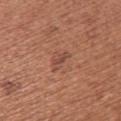patient: female, aged 48 to 52 | image-analysis metrics: a footprint of about 3.5 mm², a shape eccentricity near 0.9, and a shape-asymmetry score of about 0.4 (0 = symmetric); a lesion color around L≈48 a*≈23 b*≈28 in CIELAB, roughly 7 lightness units darker than nearby skin, and a lesion-to-skin contrast of about 6 (normalized; higher = more distinct); a border-irregularity index near 4.5/10 and a peripheral color-asymmetry measure near 0 | lesion size: ≈3 mm | image: total-body-photography crop, ~15 mm field of view | location: the back.